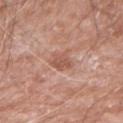The lesion was tiled from a total-body skin photograph and was not biopsied.
The subject is a male aged around 60.
Located on the right forearm.
A 15 mm close-up extracted from a 3D total-body photography capture.
Longest diameter approximately 3 mm.